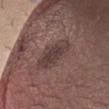<record>
<biopsy_status>not biopsied; imaged during a skin examination</biopsy_status>
</record>